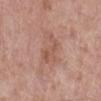| field | value |
|---|---|
| workup | no biopsy performed (imaged during a skin exam) |
| image-analysis metrics | a shape eccentricity near 0.85 and a shape-asymmetry score of about 0.45 (0 = symmetric); a mean CIELAB color near L≈54 a*≈22 b*≈28, a lesion–skin lightness drop of about 7, and a normalized lesion–skin contrast near 5; border irregularity of about 6 on a 0–10 scale, a color-variation rating of about 2.5/10, and peripheral color asymmetry of about 0.5 |
| image source | ~15 mm tile from a whole-body skin photo |
| patient | female, aged 68–72 |
| anatomic site | the left lower leg |
| size | ~3.5 mm (longest diameter) |